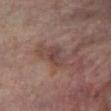Assessment:
Captured during whole-body skin photography for melanoma surveillance; the lesion was not biopsied.
Image and clinical context:
A 15 mm close-up tile from a total-body photography series done for melanoma screening. On the left lower leg. Captured under cross-polarized illumination. Longest diameter approximately 2.5 mm. Automated tile analysis of the lesion measured a mean CIELAB color near L≈40 a*≈18 b*≈20, a lesion–skin lightness drop of about 7, and a lesion-to-skin contrast of about 6 (normalized; higher = more distinct). The analysis additionally found a border-irregularity rating of about 3/10, a within-lesion color-variation index near 3/10, and peripheral color asymmetry of about 1. A male patient, aged 68 to 72.